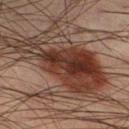Clinical summary: Automated image analysis of the tile measured an average lesion color of about L≈35 a*≈20 b*≈25 (CIELAB). And it measured a nevus-likeness score of about 100/100 and lesion-presence confidence of about 100/100. A male subject, aged 38 to 42. The tile uses cross-polarized illumination. This image is a 15 mm lesion crop taken from a total-body photograph. Located on the left lower leg. Measured at roughly 13.5 mm in maximum diameter.